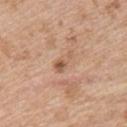  biopsy_status: not biopsied; imaged during a skin examination
  lesion_size:
    long_diameter_mm_approx: 3.0
  lighting: white-light
  site: upper back
  image:
    source: total-body photography crop
    field_of_view_mm: 15
  patient:
    sex: female
    age_approx: 45
  automated_metrics:
    cielab_L: 57
    cielab_a: 20
    cielab_b: 32
    vs_skin_contrast_norm: 6.5